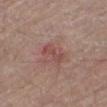follow-up: no biopsy performed (imaged during a skin exam)
subject: male, roughly 65 years of age
illumination: white-light illumination
diameter: about 3 mm
acquisition: ~15 mm crop, total-body skin-cancer survey
site: the leg